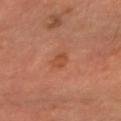Captured during whole-body skin photography for melanoma surveillance; the lesion was not biopsied. Automated image analysis of the tile measured an eccentricity of roughly 0.8. The analysis additionally found border irregularity of about 2 on a 0–10 scale, a color-variation rating of about 2/10, and a peripheral color-asymmetry measure near 0.5. And it measured a nevus-likeness score of about 25/100. The lesion is on the right forearm. This image is a 15 mm lesion crop taken from a total-body photograph. A male subject about 60 years old.